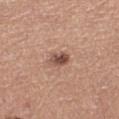Q: Was a biopsy performed?
A: total-body-photography surveillance lesion; no biopsy
Q: What did automated image analysis measure?
A: a mean CIELAB color near L≈50 a*≈21 b*≈27 and roughly 13 lightness units darker than nearby skin; border irregularity of about 2 on a 0–10 scale and peripheral color asymmetry of about 1.5; a classifier nevus-likeness of about 65/100 and lesion-presence confidence of about 100/100
Q: What is the anatomic site?
A: the left thigh
Q: Illumination type?
A: white-light
Q: Patient demographics?
A: female, aged approximately 55
Q: What is the lesion's diameter?
A: about 2.5 mm
Q: What is the imaging modality?
A: ~15 mm tile from a whole-body skin photo